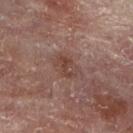Assessment: Imaged during a routine full-body skin examination; the lesion was not biopsied and no histopathology is available. Clinical summary: From the right leg. A female patient, about 80 years old. A 15 mm close-up extracted from a 3D total-body photography capture.